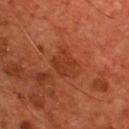Recorded during total-body skin imaging; not selected for excision or biopsy.
On the chest.
A male subject, in their mid- to late 50s.
The lesion's longest dimension is about 3.5 mm.
A region of skin cropped from a whole-body photographic capture, roughly 15 mm wide.
The tile uses cross-polarized illumination.